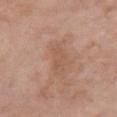* notes · total-body-photography surveillance lesion; no biopsy
* image source · 15 mm crop, total-body photography
* automated lesion analysis · an average lesion color of about L≈54 a*≈21 b*≈30 (CIELAB), a lesion–skin lightness drop of about 6, and a normalized border contrast of about 4.5; a border-irregularity index near 7.5/10, a within-lesion color-variation index near 0/10, and radial color variation of about 0
* patient · female, aged 73 to 77
* lighting · white-light illumination
* site · the front of the torso